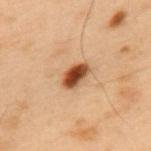Background: The patient is a male in their mid-50s. Captured under cross-polarized illumination. A region of skin cropped from a whole-body photographic capture, roughly 15 mm wide. Measured at roughly 3.5 mm in maximum diameter. The lesion is on the mid back.